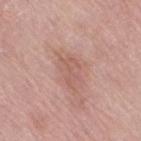The lesion was tiled from a total-body skin photograph and was not biopsied. A female subject aged 68 to 72. A roughly 15 mm field-of-view crop from a total-body skin photograph. Captured under white-light illumination. The lesion is on the right thigh.